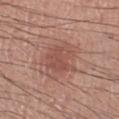Findings:
- workup — imaged on a skin check; not biopsied
- illumination — white-light illumination
- patient — male, in their 60s
- image — ~15 mm crop, total-body skin-cancer survey
- size — ≈4 mm
- body site — the right lower leg